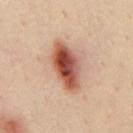Longest diameter approximately 6 mm.
A male subject aged 28 to 32.
A region of skin cropped from a whole-body photographic capture, roughly 15 mm wide.
The lesion is located on the mid back.
Histopathology of the biopsied lesion showed an atypical melanocytic neoplasm — an indeterminate (borderline) lesion.